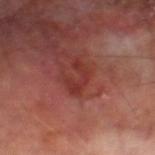<lesion>
  <biopsy_status>not biopsied; imaged during a skin examination</biopsy_status>
  <lighting>cross-polarized</lighting>
  <patient>
    <sex>male</sex>
    <age_approx>70</age_approx>
  </patient>
  <site>left thigh</site>
  <automated_metrics>
    <eccentricity>0.85</eccentricity>
    <shape_asymmetry>0.55</shape_asymmetry>
    <cielab_L>34</cielab_L>
    <cielab_a>29</cielab_a>
    <cielab_b>26</cielab_b>
    <vs_skin_darker_L>7.0</vs_skin_darker_L>
    <vs_skin_contrast_norm>6.5</vs_skin_contrast_norm>
    <nevus_likeness_0_100>0</nevus_likeness_0_100>
    <lesion_detection_confidence_0_100>100</lesion_detection_confidence_0_100>
  </automated_metrics>
  <image>
    <source>total-body photography crop</source>
    <field_of_view_mm>15</field_of_view_mm>
  </image>
</lesion>